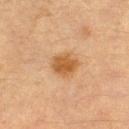Findings:
- follow-up — imaged on a skin check; not biopsied
- anatomic site — the left thigh
- acquisition — total-body-photography crop, ~15 mm field of view
- image-analysis metrics — a lesion area of about 6.5 mm² and a symmetry-axis asymmetry near 0.15; a lesion color around L≈45 a*≈19 b*≈35 in CIELAB, a lesion–skin lightness drop of about 10, and a lesion-to-skin contrast of about 8.5 (normalized; higher = more distinct)
- subject — female, in their mid-50s
- illumination — cross-polarized